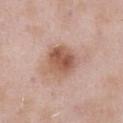biopsy status: imaged on a skin check; not biopsied | automated metrics: border irregularity of about 2 on a 0–10 scale, a color-variation rating of about 6/10, and a peripheral color-asymmetry measure near 2; a classifier nevus-likeness of about 75/100 and a detector confidence of about 100 out of 100 that the crop contains a lesion | imaging modality: ~15 mm crop, total-body skin-cancer survey | body site: the abdomen | patient: male, aged approximately 55.